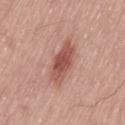Assessment: The lesion was photographed on a routine skin check and not biopsied; there is no pathology result. Image and clinical context: A male subject, roughly 75 years of age. Longest diameter approximately 5.5 mm. Located on the left thigh. The lesion-visualizer software estimated about 13 CIELAB-L* units darker than the surrounding skin. The software also gave a border-irregularity rating of about 2.5/10, internal color variation of about 3.5 on a 0–10 scale, and radial color variation of about 1. Cropped from a total-body skin-imaging series; the visible field is about 15 mm. The tile uses white-light illumination.